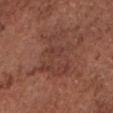<case>
  <biopsy_status>not biopsied; imaged during a skin examination</biopsy_status>
  <lesion_size>
    <long_diameter_mm_approx>6.5</long_diameter_mm_approx>
  </lesion_size>
  <automated_metrics>
    <cielab_L>40</cielab_L>
    <cielab_a>23</cielab_a>
    <cielab_b>25</cielab_b>
    <vs_skin_darker_L>6.0</vs_skin_darker_L>
    <vs_skin_contrast_norm>5.0</vs_skin_contrast_norm>
    <border_irregularity_0_10>8.0</border_irregularity_0_10>
    <color_variation_0_10>3.5</color_variation_0_10>
    <peripheral_color_asymmetry>1.0</peripheral_color_asymmetry>
  </automated_metrics>
  <image>
    <source>total-body photography crop</source>
    <field_of_view_mm>15</field_of_view_mm>
  </image>
  <patient>
    <sex>male</sex>
    <age_approx>75</age_approx>
  </patient>
  <lighting>white-light</lighting>
  <site>chest</site>
</case>